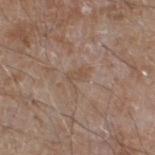image = ~15 mm crop, total-body skin-cancer survey; subject = male, in their 60s; anatomic site = the left lower leg.